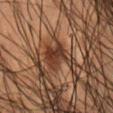Recorded during total-body skin imaging; not selected for excision or biopsy. A lesion tile, about 15 mm wide, cut from a 3D total-body photograph. Located on the lower back. A male patient, aged 53–57.